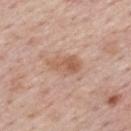Clinical impression: Imaged during a routine full-body skin examination; the lesion was not biopsied and no histopathology is available. Clinical summary: The tile uses white-light illumination. A 15 mm close-up extracted from a 3D total-body photography capture. A male patient, aged 73 to 77. Longest diameter approximately 4 mm. From the mid back.